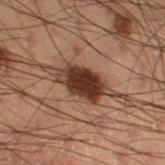Q: Is there a histopathology result?
A: catalogued during a skin exam; not biopsied
Q: Lesion location?
A: the leg
Q: What lighting was used for the tile?
A: cross-polarized illumination
Q: Who is the patient?
A: male, roughly 55 years of age
Q: How large is the lesion?
A: ~4.5 mm (longest diameter)
Q: What kind of image is this?
A: 15 mm crop, total-body photography
Q: Automated lesion metrics?
A: an average lesion color of about L≈27 a*≈18 b*≈22 (CIELAB) and about 15 CIELAB-L* units darker than the surrounding skin